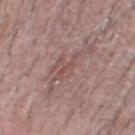Captured during whole-body skin photography for melanoma surveillance; the lesion was not biopsied. The lesion is located on the head or neck. Longest diameter approximately 6.5 mm. This is a white-light tile. A region of skin cropped from a whole-body photographic capture, roughly 15 mm wide. The patient is a male roughly 60 years of age.